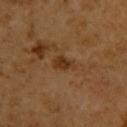follow-up — catalogued during a skin exam; not biopsied
TBP lesion metrics — an area of roughly 4 mm², an eccentricity of roughly 0.75, and a symmetry-axis asymmetry near 0.35; a within-lesion color-variation index near 2.5/10 and radial color variation of about 1; a classifier nevus-likeness of about 20/100 and lesion-presence confidence of about 100/100
subject — female, aged 53 to 57
image — total-body-photography crop, ~15 mm field of view
location — the upper back
lighting — cross-polarized illumination